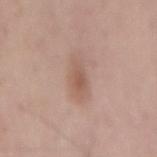Imaged during a routine full-body skin examination; the lesion was not biopsied and no histopathology is available. A male subject approximately 55 years of age. The lesion is on the mid back. An algorithmic analysis of the crop reported a lesion color around L≈57 a*≈19 b*≈27 in CIELAB, a lesion–skin lightness drop of about 8, and a normalized border contrast of about 6. About 5 mm across. Cropped from a whole-body photographic skin survey; the tile spans about 15 mm. This is a white-light tile.